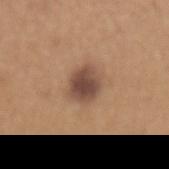Q: Was a biopsy performed?
A: no biopsy performed (imaged during a skin exam)
Q: What did automated image analysis measure?
A: an area of roughly 8.5 mm²; a border-irregularity rating of about 1.5/10, a color-variation rating of about 3.5/10, and a peripheral color-asymmetry measure near 1; a nevus-likeness score of about 65/100 and a detector confidence of about 100 out of 100 that the crop contains a lesion
Q: Who is the patient?
A: female, aged 33 to 37
Q: How was the tile lit?
A: white-light
Q: What is the lesion's diameter?
A: about 3.5 mm
Q: How was this image acquired?
A: ~15 mm tile from a whole-body skin photo
Q: Lesion location?
A: the mid back